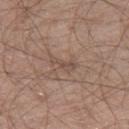{"biopsy_status": "not biopsied; imaged during a skin examination", "automated_metrics": {"area_mm2_approx": 4.5, "eccentricity": 0.9, "cielab_L": 50, "cielab_a": 16, "cielab_b": 24, "lesion_detection_confidence_0_100": 55}, "patient": {"sex": "male", "age_approx": 60}, "site": "right thigh", "image": {"source": "total-body photography crop", "field_of_view_mm": 15}, "lighting": "white-light"}